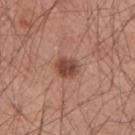Clinical impression:
No biopsy was performed on this lesion — it was imaged during a full skin examination and was not determined to be concerning.
Image and clinical context:
The tile uses white-light illumination. The patient is a male approximately 60 years of age. About 2.5 mm across. A region of skin cropped from a whole-body photographic capture, roughly 15 mm wide. The lesion is located on the arm.